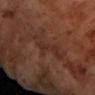Captured under cross-polarized illumination. Located on the arm. Cropped from a total-body skin-imaging series; the visible field is about 15 mm. The subject is a female aged 68 to 72. Automated image analysis of the tile measured a lesion color around L≈28 a*≈20 b*≈24 in CIELAB, a lesion–skin lightness drop of about 5, and a normalized lesion–skin contrast near 5.5. It also reported a border-irregularity index near 7/10, a color-variation rating of about 1/10, and radial color variation of about 0.5.